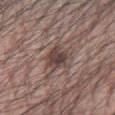Findings:
* location — the left forearm
* imaging modality — 15 mm crop, total-body photography
* subject — male, aged 68–72